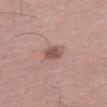Impression:
No biopsy was performed on this lesion — it was imaged during a full skin examination and was not determined to be concerning.